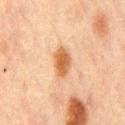Impression: Recorded during total-body skin imaging; not selected for excision or biopsy. Background: On the mid back. The total-body-photography lesion software estimated a classifier nevus-likeness of about 95/100. The subject is a male in their mid- to late 60s. This image is a 15 mm lesion crop taken from a total-body photograph. The tile uses cross-polarized illumination. Approximately 3.5 mm at its widest.